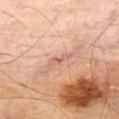- notes: catalogued during a skin exam; not biopsied
- acquisition: 15 mm crop, total-body photography
- lighting: cross-polarized
- location: the left thigh
- patient: male, about 70 years old
- size: ~3 mm (longest diameter)